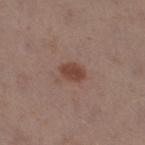  biopsy_status: not biopsied; imaged during a skin examination
  lesion_size:
    long_diameter_mm_approx: 3.0
  patient:
    sex: female
    age_approx: 40
  site: right thigh
  image:
    source: total-body photography crop
    field_of_view_mm: 15